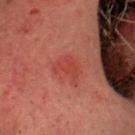Q: Was this lesion biopsied?
A: no biopsy performed (imaged during a skin exam)
Q: How was this image acquired?
A: total-body-photography crop, ~15 mm field of view
Q: Where on the body is the lesion?
A: the head or neck
Q: Who is the patient?
A: male, roughly 50 years of age
Q: What lighting was used for the tile?
A: cross-polarized